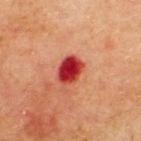biopsy status: catalogued during a skin exam; not biopsied
subject: male, aged approximately 70
image source: ~15 mm tile from a whole-body skin photo
lesion diameter: ~3.5 mm (longest diameter)
TBP lesion metrics: a footprint of about 6.5 mm², an outline eccentricity of about 0.65 (0 = round, 1 = elongated), and a shape-asymmetry score of about 0.15 (0 = symmetric); a mean CIELAB color near L≈34 a*≈39 b*≈29, about 17 CIELAB-L* units darker than the surrounding skin, and a lesion-to-skin contrast of about 14 (normalized; higher = more distinct); a border-irregularity rating of about 1/10 and a within-lesion color-variation index near 4/10
anatomic site: the upper back